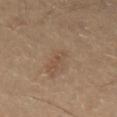follow-up: total-body-photography surveillance lesion; no biopsy | subject: male, aged 53–57 | acquisition: 15 mm crop, total-body photography | lesion size: ≈1 mm | body site: the abdomen | illumination: cross-polarized.